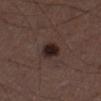No biopsy was performed on this lesion — it was imaged during a full skin examination and was not determined to be concerning. Longest diameter approximately 3 mm. This image is a 15 mm lesion crop taken from a total-body photograph. The patient is a male aged around 50. Imaged with white-light lighting. The lesion is on the left thigh.